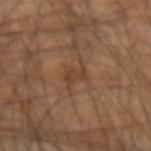workup — imaged on a skin check; not biopsied | illumination — cross-polarized | site — the left forearm | acquisition — total-body-photography crop, ~15 mm field of view | subject — male, roughly 65 years of age | TBP lesion metrics — a shape eccentricity near 0.85 and a symmetry-axis asymmetry near 0.45; a lesion color around L≈38 a*≈19 b*≈29 in CIELAB, about 6 CIELAB-L* units darker than the surrounding skin, and a lesion-to-skin contrast of about 6 (normalized; higher = more distinct); a border-irregularity rating of about 5/10 and peripheral color asymmetry of about 0.